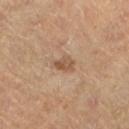body site = the left lower leg
subject = female, aged around 60
acquisition = total-body-photography crop, ~15 mm field of view
lesion size = ≈2.5 mm
TBP lesion metrics = an average lesion color of about L≈48 a*≈17 b*≈29 (CIELAB), a lesion–skin lightness drop of about 9, and a normalized border contrast of about 7; a classifier nevus-likeness of about 0/100 and a lesion-detection confidence of about 100/100
lighting = cross-polarized illumination